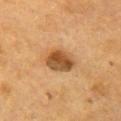Notes:
• workup · imaged on a skin check; not biopsied
• lighting · cross-polarized
• anatomic site · the chest
• patient · female, aged around 55
• acquisition · 15 mm crop, total-body photography